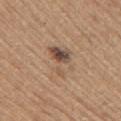No biopsy was performed on this lesion — it was imaged during a full skin examination and was not determined to be concerning. A male subject in their mid- to late 60s. The lesion is located on the right upper arm. This is a white-light tile. This image is a 15 mm lesion crop taken from a total-body photograph. The lesion's longest dimension is about 4.5 mm.